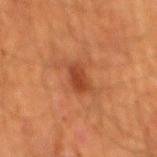The lesion was photographed on a routine skin check and not biopsied; there is no pathology result.